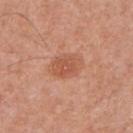workup = no biopsy performed (imaged during a skin exam)
lighting = white-light
patient = male, in their mid-50s
imaging modality = ~15 mm tile from a whole-body skin photo
image-analysis metrics = a border-irregularity index near 1.5/10, a color-variation rating of about 3/10, and radial color variation of about 1
site = the chest